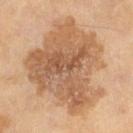image source: ~15 mm tile from a whole-body skin photo
location: the left leg
patient: male, aged approximately 65